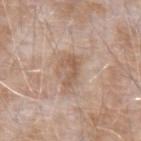{
  "biopsy_status": "not biopsied; imaged during a skin examination",
  "lighting": "white-light",
  "patient": {
    "sex": "male",
    "age_approx": 75
  },
  "site": "left forearm",
  "image": {
    "source": "total-body photography crop",
    "field_of_view_mm": 15
  }
}